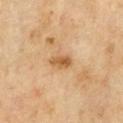Q: Was this lesion biopsied?
A: catalogued during a skin exam; not biopsied
Q: Who is the patient?
A: male, aged 68–72
Q: Where on the body is the lesion?
A: the chest
Q: What kind of image is this?
A: 15 mm crop, total-body photography